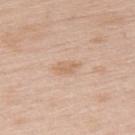The lesion-visualizer software estimated a lesion area of about 4 mm² and a shape-asymmetry score of about 0.35 (0 = symmetric). The analysis additionally found an average lesion color of about L≈65 a*≈18 b*≈32 (CIELAB), a lesion–skin lightness drop of about 7, and a normalized lesion–skin contrast near 5.5. The software also gave a border-irregularity rating of about 3.5/10 and a color-variation rating of about 1.5/10. The patient is a female about 65 years old. A 15 mm close-up tile from a total-body photography series done for melanoma screening. The recorded lesion diameter is about 3 mm. The lesion is on the upper back.